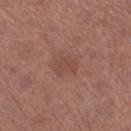<record>
  <biopsy_status>not biopsied; imaged during a skin examination</biopsy_status>
</record>